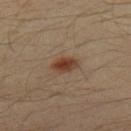{"biopsy_status": "not biopsied; imaged during a skin examination", "patient": {"sex": "male", "age_approx": 30}, "image": {"source": "total-body photography crop", "field_of_view_mm": 15}, "site": "leg"}